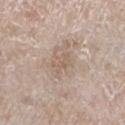Captured during whole-body skin photography for melanoma surveillance; the lesion was not biopsied. The tile uses white-light illumination. A region of skin cropped from a whole-body photographic capture, roughly 15 mm wide. Approximately 3 mm at its widest. A female subject roughly 75 years of age. Located on the left lower leg. An algorithmic analysis of the crop reported internal color variation of about 0.5 on a 0–10 scale and a peripheral color-asymmetry measure near 0.